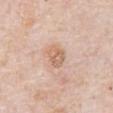Assessment: No biopsy was performed on this lesion — it was imaged during a full skin examination and was not determined to be concerning. Acquisition and patient details: Imaged with white-light lighting. From the abdomen. Measured at roughly 3 mm in maximum diameter. The patient is a male aged 78 to 82. A roughly 15 mm field-of-view crop from a total-body skin photograph. The total-body-photography lesion software estimated a lesion area of about 6.5 mm² and an eccentricity of roughly 0.6.